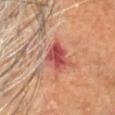No biopsy was performed on this lesion — it was imaged during a full skin examination and was not determined to be concerning.